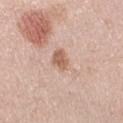biopsy status: no biopsy performed (imaged during a skin exam); tile lighting: white-light illumination; image: 15 mm crop, total-body photography; lesion diameter: ≈4 mm; anatomic site: the right upper arm; image-analysis metrics: a border-irregularity rating of about 4/10, internal color variation of about 3 on a 0–10 scale, and a peripheral color-asymmetry measure near 1; patient: female, aged 38–42.